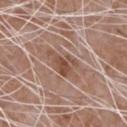The lesion was tiled from a total-body skin photograph and was not biopsied.
Located on the upper back.
About 4 mm across.
An algorithmic analysis of the crop reported a border-irregularity index near 6.5/10, a within-lesion color-variation index near 3.5/10, and peripheral color asymmetry of about 1.
A male subject, aged 58 to 62.
A 15 mm close-up tile from a total-body photography series done for melanoma screening.